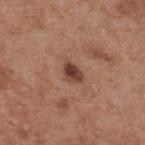  biopsy_status: not biopsied; imaged during a skin examination
  lighting: white-light
  site: back
  image:
    source: total-body photography crop
    field_of_view_mm: 15
  patient:
    sex: male
    age_approx: 55
  automated_metrics:
    area_mm2_approx: 3.5
    eccentricity: 0.75
    cielab_L: 41
    cielab_a: 21
    cielab_b: 26
    vs_skin_darker_L: 13.0
    vs_skin_contrast_norm: 10.5
    color_variation_0_10: 4.0
  lesion_size:
    long_diameter_mm_approx: 2.5